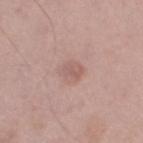Q: Is there a histopathology result?
A: imaged on a skin check; not biopsied
Q: Automated lesion metrics?
A: a mean CIELAB color near L≈59 a*≈19 b*≈23, a lesion–skin lightness drop of about 7, and a lesion-to-skin contrast of about 4.5 (normalized; higher = more distinct); border irregularity of about 2.5 on a 0–10 scale, internal color variation of about 2.5 on a 0–10 scale, and radial color variation of about 1
Q: Who is the patient?
A: male, aged 68–72
Q: How was this image acquired?
A: total-body-photography crop, ~15 mm field of view
Q: Lesion size?
A: ~3 mm (longest diameter)
Q: What is the anatomic site?
A: the left thigh
Q: How was the tile lit?
A: white-light illumination